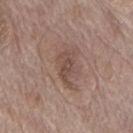Findings:
* notes: no biopsy performed (imaged during a skin exam)
* subject: male, aged 68–72
* imaging modality: ~15 mm crop, total-body skin-cancer survey
* TBP lesion metrics: a lesion area of about 6 mm², an eccentricity of roughly 0.9, and two-axis asymmetry of about 0.3; a border-irregularity index near 4/10, a within-lesion color-variation index near 2.5/10, and a peripheral color-asymmetry measure near 1; a nevus-likeness score of about 0/100 and a lesion-detection confidence of about 100/100
* body site: the mid back
* lesion diameter: ~4.5 mm (longest diameter)
* lighting: white-light illumination